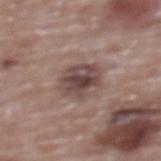Imaged during a routine full-body skin examination; the lesion was not biopsied and no histopathology is available.
The lesion's longest dimension is about 4.5 mm.
A female patient approximately 65 years of age.
A 15 mm crop from a total-body photograph taken for skin-cancer surveillance.
Located on the upper back.